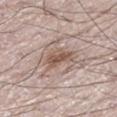Part of a total-body skin-imaging series; this lesion was reviewed on a skin check and was not flagged for biopsy. Cropped from a total-body skin-imaging series; the visible field is about 15 mm. Located on the right leg. The patient is a male aged 68–72. The recorded lesion diameter is about 4 mm.